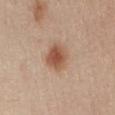This lesion was catalogued during total-body skin photography and was not selected for biopsy. Measured at roughly 3.5 mm in maximum diameter. Cropped from a total-body skin-imaging series; the visible field is about 15 mm. From the front of the torso. A male patient aged 63 to 67. The total-body-photography lesion software estimated a classifier nevus-likeness of about 100/100 and a detector confidence of about 100 out of 100 that the crop contains a lesion.